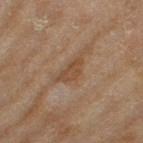Case summary:
* biopsy status · catalogued during a skin exam; not biopsied
* patient · female, about 60 years old
* location · the left thigh
* imaging modality · 15 mm crop, total-body photography